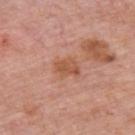Captured during whole-body skin photography for melanoma surveillance; the lesion was not biopsied.
The lesion is on the upper back.
Imaged with white-light lighting.
Measured at roughly 3 mm in maximum diameter.
The total-body-photography lesion software estimated a shape eccentricity near 0.8 and a shape-asymmetry score of about 0.3 (0 = symmetric). And it measured a border-irregularity rating of about 3/10 and peripheral color asymmetry of about 0.5.
A 15 mm crop from a total-body photograph taken for skin-cancer surveillance.
The patient is a male about 75 years old.